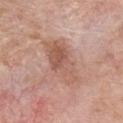Q: Is there a histopathology result?
A: no biopsy performed (imaged during a skin exam)
Q: Lesion location?
A: the chest
Q: How was the tile lit?
A: white-light
Q: How large is the lesion?
A: ~6.5 mm (longest diameter)
Q: What kind of image is this?
A: total-body-photography crop, ~15 mm field of view
Q: What are the patient's age and sex?
A: male, aged 58 to 62